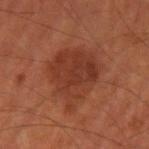notes: total-body-photography surveillance lesion; no biopsy
tile lighting: cross-polarized
image-analysis metrics: an area of roughly 25 mm², a shape eccentricity near 0.55, and two-axis asymmetry of about 0.3; an average lesion color of about L≈34 a*≈25 b*≈29 (CIELAB) and a normalized border contrast of about 7; border irregularity of about 3.5 on a 0–10 scale, internal color variation of about 3.5 on a 0–10 scale, and radial color variation of about 1; a classifier nevus-likeness of about 85/100 and a lesion-detection confidence of about 100/100
imaging modality: total-body-photography crop, ~15 mm field of view
anatomic site: the left upper arm
subject: male, aged 53 to 57
lesion size: ~7 mm (longest diameter)